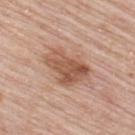Part of a total-body skin-imaging series; this lesion was reviewed on a skin check and was not flagged for biopsy.
A 15 mm close-up tile from a total-body photography series done for melanoma screening.
An algorithmic analysis of the crop reported an outline eccentricity of about 0.75 (0 = round, 1 = elongated). The software also gave a lesion color around L≈55 a*≈21 b*≈30 in CIELAB, roughly 11 lightness units darker than nearby skin, and a normalized border contrast of about 8. It also reported a border-irregularity rating of about 3.5/10, internal color variation of about 5.5 on a 0–10 scale, and peripheral color asymmetry of about 2. The analysis additionally found a classifier nevus-likeness of about 55/100 and a lesion-detection confidence of about 100/100.
A male subject, about 80 years old.
Located on the back.